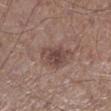{"lighting": "white-light", "patient": {"sex": "male", "age_approx": 60}, "image": {"source": "total-body photography crop", "field_of_view_mm": 15}, "automated_metrics": {"area_mm2_approx": 9.0}, "site": "left lower leg"}